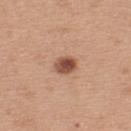site = the upper back | patient = female, in their 40s | illumination = white-light illumination | image source = total-body-photography crop, ~15 mm field of view | diameter = ≈3 mm.